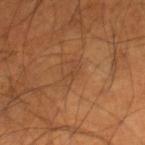Q: Was this lesion biopsied?
A: imaged on a skin check; not biopsied
Q: What is the anatomic site?
A: the leg
Q: Patient demographics?
A: male, in their mid-50s
Q: How large is the lesion?
A: about 3 mm
Q: What did automated image analysis measure?
A: an outline eccentricity of about 0.9 (0 = round, 1 = elongated) and two-axis asymmetry of about 0.35; a normalized lesion–skin contrast near 4.5; radial color variation of about 0
Q: What lighting was used for the tile?
A: cross-polarized
Q: What kind of image is this?
A: ~15 mm tile from a whole-body skin photo